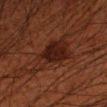Assessment:
Imaged during a routine full-body skin examination; the lesion was not biopsied and no histopathology is available.
Context:
Longest diameter approximately 6.5 mm. A 15 mm crop from a total-body photograph taken for skin-cancer surveillance. The lesion is on the left upper arm. A male patient, aged 48 to 52. Captured under cross-polarized illumination. The total-body-photography lesion software estimated an area of roughly 16 mm² and an outline eccentricity of about 0.85 (0 = round, 1 = elongated). It also reported internal color variation of about 3.5 on a 0–10 scale and radial color variation of about 1. The software also gave an automated nevus-likeness rating near 95 out of 100 and lesion-presence confidence of about 100/100.